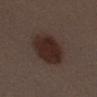follow-up — catalogued during a skin exam; not biopsied | image source — ~15 mm crop, total-body skin-cancer survey | size — about 5.5 mm | lighting — white-light illumination | anatomic site — the mid back | automated lesion analysis — a detector confidence of about 100 out of 100 that the crop contains a lesion | patient — female, approximately 50 years of age.